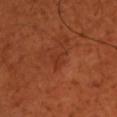* notes · imaged on a skin check; not biopsied
* TBP lesion metrics · an area of roughly 3.5 mm² and a shape eccentricity near 0.65; an average lesion color of about L≈35 a*≈30 b*≈33 (CIELAB) and a normalized lesion–skin contrast near 4.5
* patient · male, in their 50s
* anatomic site · the left upper arm
* tile lighting · cross-polarized
* imaging modality · ~15 mm tile from a whole-body skin photo